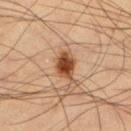tile lighting — cross-polarized
subject — male, aged approximately 35
size — about 3 mm
anatomic site — the left lower leg
image source — ~15 mm crop, total-body skin-cancer survey